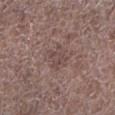Q: Was this lesion biopsied?
A: imaged on a skin check; not biopsied
Q: What did automated image analysis measure?
A: a shape eccentricity near 0.55 and a symmetry-axis asymmetry near 0.25; an automated nevus-likeness rating near 0 out of 100
Q: What kind of image is this?
A: 15 mm crop, total-body photography
Q: Illumination type?
A: white-light
Q: Who is the patient?
A: male, aged 68 to 72
Q: Where on the body is the lesion?
A: the leg